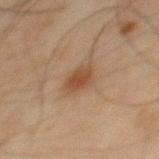Case summary:
* follow-up — catalogued during a skin exam; not biopsied
* subject — male, aged 43 to 47
* acquisition — total-body-photography crop, ~15 mm field of view
* TBP lesion metrics — a mean CIELAB color near L≈38 a*≈16 b*≈27, roughly 8 lightness units darker than nearby skin, and a normalized lesion–skin contrast near 7.5; a border-irregularity rating of about 2/10, a color-variation rating of about 2.5/10, and peripheral color asymmetry of about 0.5; a detector confidence of about 100 out of 100 that the crop contains a lesion
* lighting — cross-polarized illumination
* site — the back
* diameter — ≈3 mm